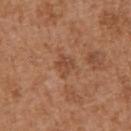No biopsy was performed on this lesion — it was imaged during a full skin examination and was not determined to be concerning. Automated tile analysis of the lesion measured an average lesion color of about L≈47 a*≈23 b*≈32 (CIELAB), about 7 CIELAB-L* units darker than the surrounding skin, and a normalized lesion–skin contrast near 5.5. The software also gave a border-irregularity index near 3/10, a within-lesion color-variation index near 3/10, and a peripheral color-asymmetry measure near 1. The software also gave a nevus-likeness score of about 0/100. A female patient aged 38–42. A roughly 15 mm field-of-view crop from a total-body skin photograph. Located on the arm. The lesion's longest dimension is about 2.5 mm.